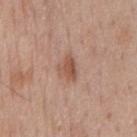subject=male, roughly 60 years of age; acquisition=~15 mm tile from a whole-body skin photo; site=the chest; lesion size=about 3 mm; tile lighting=white-light.